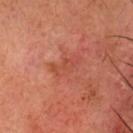- notes — total-body-photography surveillance lesion; no biopsy
- imaging modality — 15 mm crop, total-body photography
- tile lighting — cross-polarized illumination
- diameter — about 4 mm
- subject — male, aged approximately 65
- automated metrics — a shape-asymmetry score of about 0.35 (0 = symmetric); a lesion color around L≈49 a*≈31 b*≈33 in CIELAB and a lesion-to-skin contrast of about 4.5 (normalized; higher = more distinct); border irregularity of about 4.5 on a 0–10 scale and internal color variation of about 2.5 on a 0–10 scale; a lesion-detection confidence of about 100/100
- anatomic site — the head or neck